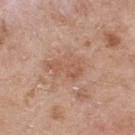Captured during whole-body skin photography for melanoma surveillance; the lesion was not biopsied. The subject is a male approximately 55 years of age. The lesion-visualizer software estimated a border-irregularity rating of about 4.5/10, a within-lesion color-variation index near 3/10, and radial color variation of about 1. And it measured a nevus-likeness score of about 0/100. The tile uses white-light illumination. Longest diameter approximately 4.5 mm. A roughly 15 mm field-of-view crop from a total-body skin photograph. On the upper back.